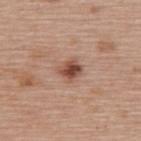| field | value |
|---|---|
| follow-up | imaged on a skin check; not biopsied |
| anatomic site | the back |
| lesion diameter | ≈2.5 mm |
| tile lighting | white-light illumination |
| subject | female, in their 60s |
| imaging modality | 15 mm crop, total-body photography |
| image-analysis metrics | a lesion area of about 5.5 mm², an eccentricity of roughly 0.45, and two-axis asymmetry of about 0.2; an average lesion color of about L≈49 a*≈22 b*≈28 (CIELAB), roughly 13 lightness units darker than nearby skin, and a normalized border contrast of about 9 |